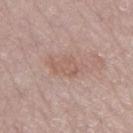follow-up: no biopsy performed (imaged during a skin exam)
tile lighting: white-light
body site: the left thigh
subject: female, roughly 70 years of age
diameter: ~4.5 mm (longest diameter)
image: ~15 mm crop, total-body skin-cancer survey
automated lesion analysis: a lesion area of about 7.5 mm² and an outline eccentricity of about 0.8 (0 = round, 1 = elongated); a lesion color around L≈58 a*≈18 b*≈25 in CIELAB and a normalized border contrast of about 5